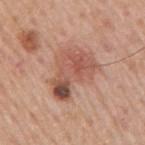{
  "biopsy_status": "not biopsied; imaged during a skin examination",
  "patient": {
    "sex": "male",
    "age_approx": 75
  },
  "image": {
    "source": "total-body photography crop",
    "field_of_view_mm": 15
  },
  "site": "right upper arm"
}